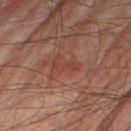Impression:
No biopsy was performed on this lesion — it was imaged during a full skin examination and was not determined to be concerning.
Background:
The lesion is located on the right thigh. A male patient, roughly 75 years of age. A region of skin cropped from a whole-body photographic capture, roughly 15 mm wide. The lesion-visualizer software estimated a mean CIELAB color near L≈35 a*≈22 b*≈23. The analysis additionally found a border-irregularity index near 6.5/10, a color-variation rating of about 0/10, and radial color variation of about 0. About 3.5 mm across. This is a cross-polarized tile.